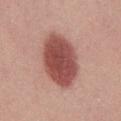follow-up: imaged on a skin check; not biopsied | diameter: ~7 mm (longest diameter) | TBP lesion metrics: an area of roughly 24 mm², an eccentricity of roughly 0.8, and a shape-asymmetry score of about 0.1 (0 = symmetric); a lesion color around L≈50 a*≈27 b*≈25 in CIELAB, a lesion–skin lightness drop of about 16, and a lesion-to-skin contrast of about 11 (normalized; higher = more distinct); a border-irregularity rating of about 1.5/10 and a within-lesion color-variation index near 3.5/10; a detector confidence of about 100 out of 100 that the crop contains a lesion | imaging modality: total-body-photography crop, ~15 mm field of view | illumination: white-light | subject: male, about 30 years old.